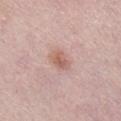Part of a total-body skin-imaging series; this lesion was reviewed on a skin check and was not flagged for biopsy. Captured under white-light illumination. An algorithmic analysis of the crop reported an area of roughly 4.5 mm² and a symmetry-axis asymmetry near 0.3. And it measured border irregularity of about 2.5 on a 0–10 scale, a color-variation rating of about 3.5/10, and radial color variation of about 1. It also reported a classifier nevus-likeness of about 65/100 and a lesion-detection confidence of about 100/100. About 3 mm across. A roughly 15 mm field-of-view crop from a total-body skin photograph. The lesion is located on the right lower leg. The patient is a female aged approximately 65.